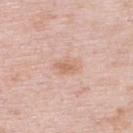| feature | finding |
|---|---|
| follow-up | total-body-photography surveillance lesion; no biopsy |
| tile lighting | white-light illumination |
| anatomic site | the upper back |
| imaging modality | 15 mm crop, total-body photography |
| subject | female, aged approximately 65 |
| size | ~3 mm (longest diameter) |